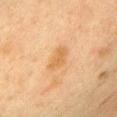The lesion was tiled from a total-body skin photograph and was not biopsied.
The recorded lesion diameter is about 3.5 mm.
The patient is a female about 40 years old.
The lesion is on the chest.
This image is a 15 mm lesion crop taken from a total-body photograph.
Automated image analysis of the tile measured an area of roughly 5.5 mm², a shape eccentricity near 0.85, and two-axis asymmetry of about 0.25. And it measured a lesion color around L≈54 a*≈18 b*≈37 in CIELAB, roughly 7 lightness units darker than nearby skin, and a normalized lesion–skin contrast near 6.